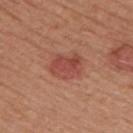follow-up — imaged on a skin check; not biopsied
site — the upper back
automated metrics — a mean CIELAB color near L≈48 a*≈28 b*≈28 and roughly 9 lightness units darker than nearby skin
lighting — white-light illumination
imaging modality — ~15 mm crop, total-body skin-cancer survey
subject — male, aged around 55
lesion size — ~4 mm (longest diameter)